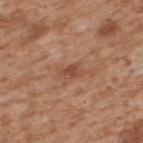| feature | finding |
|---|---|
| biopsy status | no biopsy performed (imaged during a skin exam) |
| tile lighting | white-light |
| site | the upper back |
| subject | male, aged approximately 65 |
| automated lesion analysis | a lesion area of about 3.5 mm², an outline eccentricity of about 0.8 (0 = round, 1 = elongated), and a shape-asymmetry score of about 0.3 (0 = symmetric); a mean CIELAB color near L≈48 a*≈23 b*≈32, a lesion–skin lightness drop of about 9, and a normalized border contrast of about 6.5; border irregularity of about 3 on a 0–10 scale and radial color variation of about 0 |
| imaging modality | ~15 mm crop, total-body skin-cancer survey |
| diameter | ≈2.5 mm |